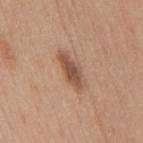Assessment:
This lesion was catalogued during total-body skin photography and was not selected for biopsy.
Image and clinical context:
Automated image analysis of the tile measured an area of roughly 7 mm². It also reported a normalized lesion–skin contrast near 8.5. It also reported a peripheral color-asymmetry measure near 1. The analysis additionally found a nevus-likeness score of about 90/100 and a lesion-detection confidence of about 100/100. A roughly 15 mm field-of-view crop from a total-body skin photograph. Located on the mid back. The lesion's longest dimension is about 5 mm. Captured under white-light illumination. The patient is a male aged approximately 30.